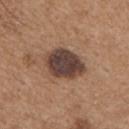  biopsy_status: not biopsied; imaged during a skin examination
  patient:
    sex: male
    age_approx: 65
  site: chest
  image:
    source: total-body photography crop
    field_of_view_mm: 15